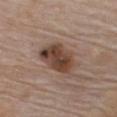{"biopsy_status": "not biopsied; imaged during a skin examination", "patient": {"sex": "male", "age_approx": 75}, "site": "chest", "image": {"source": "total-body photography crop", "field_of_view_mm": 15}}